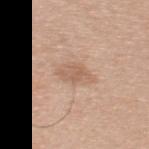Clinical impression: The lesion was tiled from a total-body skin photograph and was not biopsied. Clinical summary: From the back. This is a white-light tile. A 15 mm close-up extracted from a 3D total-body photography capture. Approximately 4 mm at its widest. The subject is a male aged around 45.